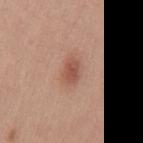<tbp_lesion>
<image>
  <source>total-body photography crop</source>
  <field_of_view_mm>15</field_of_view_mm>
</image>
<site>mid back</site>
<patient>
  <sex>male</sex>
  <age_approx>30</age_approx>
</patient>
</tbp_lesion>